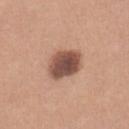{"biopsy_status": "not biopsied; imaged during a skin examination", "image": {"source": "total-body photography crop", "field_of_view_mm": 15}, "site": "right lower leg", "lesion_size": {"long_diameter_mm_approx": 4.0}, "patient": {"sex": "female", "age_approx": 45}, "lighting": "white-light"}